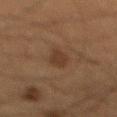This lesion was catalogued during total-body skin photography and was not selected for biopsy. This image is a 15 mm lesion crop taken from a total-body photograph. The lesion's longest dimension is about 3.5 mm. The lesion is located on the mid back. This is a cross-polarized tile. A male subject in their mid- to late 60s.